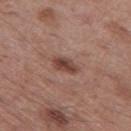Q: Is there a histopathology result?
A: imaged on a skin check; not biopsied
Q: Automated lesion metrics?
A: a footprint of about 4.5 mm², an outline eccentricity of about 0.85 (0 = round, 1 = elongated), and two-axis asymmetry of about 0.15; a within-lesion color-variation index near 2.5/10 and peripheral color asymmetry of about 1
Q: What is the imaging modality?
A: ~15 mm tile from a whole-body skin photo
Q: What is the anatomic site?
A: the leg
Q: Who is the patient?
A: male, about 55 years old